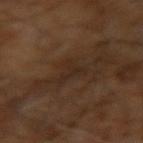  biopsy_status: not biopsied; imaged during a skin examination
  patient:
    sex: male
    age_approx: 60
  lesion_size:
    long_diameter_mm_approx: 3.0
  image:
    source: total-body photography crop
    field_of_view_mm: 15
  lighting: cross-polarized
  site: right arm